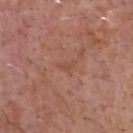biopsy status — imaged on a skin check; not biopsied | TBP lesion metrics — an area of roughly 2 mm² and a symmetry-axis asymmetry near 0.55; about 5 CIELAB-L* units darker than the surrounding skin and a lesion-to-skin contrast of about 4.5 (normalized; higher = more distinct); a border-irregularity rating of about 5.5/10 and radial color variation of about 0; a nevus-likeness score of about 0/100 and lesion-presence confidence of about 90/100 | image source — 15 mm crop, total-body photography | site — the head or neck | subject — male, aged approximately 60 | illumination — white-light illumination.